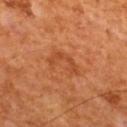biopsy status = catalogued during a skin exam; not biopsied | subject = male, aged 63 to 67 | location = the mid back | imaging modality = 15 mm crop, total-body photography | lighting = cross-polarized | diameter = about 4.5 mm.